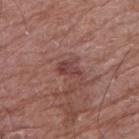| field | value |
|---|---|
| follow-up | catalogued during a skin exam; not biopsied |
| location | the arm |
| patient | male, aged 73 to 77 |
| lesion diameter | ~3 mm (longest diameter) |
| tile lighting | white-light |
| automated metrics | a footprint of about 4 mm², an outline eccentricity of about 0.85 (0 = round, 1 = elongated), and a shape-asymmetry score of about 0.4 (0 = symmetric); a mean CIELAB color near L≈42 a*≈22 b*≈22, about 9 CIELAB-L* units darker than the surrounding skin, and a lesion-to-skin contrast of about 7 (normalized; higher = more distinct); border irregularity of about 4 on a 0–10 scale and a within-lesion color-variation index near 2.5/10; an automated nevus-likeness rating near 0 out of 100 |
| image | ~15 mm crop, total-body skin-cancer survey |